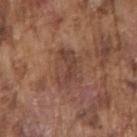No biopsy was performed on this lesion — it was imaged during a full skin examination and was not determined to be concerning.
From the right upper arm.
A lesion tile, about 15 mm wide, cut from a 3D total-body photograph.
About 5 mm across.
This is a white-light tile.
A male patient, in their mid- to late 70s.